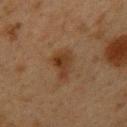Imaged during a routine full-body skin examination; the lesion was not biopsied and no histopathology is available. A region of skin cropped from a whole-body photographic capture, roughly 15 mm wide. From the back. A female patient aged 38–42.